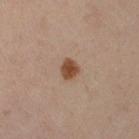Clinical impression: Imaged during a routine full-body skin examination; the lesion was not biopsied and no histopathology is available. Acquisition and patient details: The lesion is located on the right lower leg. The patient is a female approximately 40 years of age. The tile uses cross-polarized illumination. A 15 mm crop from a total-body photograph taken for skin-cancer surveillance. An algorithmic analysis of the crop reported an average lesion color of about L≈46 a*≈20 b*≈31 (CIELAB) and a normalized border contrast of about 10.5. The software also gave an automated nevus-likeness rating near 100 out of 100 and a lesion-detection confidence of about 100/100. The lesion's longest dimension is about 2 mm.